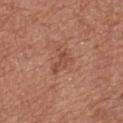Impression:
The lesion was tiled from a total-body skin photograph and was not biopsied.
Image and clinical context:
Cropped from a total-body skin-imaging series; the visible field is about 15 mm. Longest diameter approximately 3 mm. The lesion is on the upper back. Imaged with white-light lighting. A female patient in their 60s.